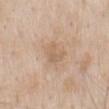About 2.5 mm across. A male subject, approximately 60 years of age. On the mid back. A roughly 15 mm field-of-view crop from a total-body skin photograph. Captured under white-light illumination.